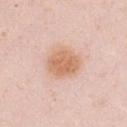biopsy_status: not biopsied; imaged during a skin examination
site: chest
lighting: white-light
patient:
  sex: female
  age_approx: 30
lesion_size:
  long_diameter_mm_approx: 4.0
image:
  source: total-body photography crop
  field_of_view_mm: 15